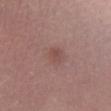follow-up = catalogued during a skin exam; not biopsied
body site = the right lower leg
patient = female, roughly 65 years of age
imaging modality = 15 mm crop, total-body photography